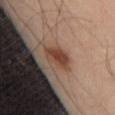The lesion was tiled from a total-body skin photograph and was not biopsied.
A male subject, roughly 45 years of age.
The lesion-visualizer software estimated an area of roughly 7 mm². It also reported an average lesion color of about L≈42 a*≈20 b*≈27 (CIELAB) and about 7 CIELAB-L* units darker than the surrounding skin. The analysis additionally found a border-irregularity index near 2.5/10, a color-variation rating of about 3/10, and radial color variation of about 1. The software also gave a nevus-likeness score of about 80/100.
This is a cross-polarized tile.
On the abdomen.
The recorded lesion diameter is about 4 mm.
This image is a 15 mm lesion crop taken from a total-body photograph.